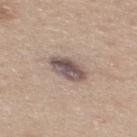Q: Was this lesion biopsied?
A: imaged on a skin check; not biopsied
Q: What did automated image analysis measure?
A: a lesion area of about 9 mm², an eccentricity of roughly 0.75, and two-axis asymmetry of about 0.1
Q: What is the imaging modality?
A: 15 mm crop, total-body photography
Q: Who is the patient?
A: male, in their mid- to late 30s
Q: What is the anatomic site?
A: the mid back
Q: What lighting was used for the tile?
A: white-light illumination